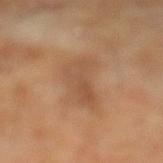<record>
<biopsy_status>not biopsied; imaged during a skin examination</biopsy_status>
<lighting>cross-polarized</lighting>
<site>left lower leg</site>
<lesion_size>
  <long_diameter_mm_approx>5.5</long_diameter_mm_approx>
</lesion_size>
<image>
  <source>total-body photography crop</source>
  <field_of_view_mm>15</field_of_view_mm>
</image>
<automated_metrics>
  <area_mm2_approx>10.0</area_mm2_approx>
  <eccentricity>0.9</eccentricity>
  <shape_asymmetry>0.4</shape_asymmetry>
  <vs_skin_contrast_norm>5.0</vs_skin_contrast_norm>
</automated_metrics>
<patient>
  <sex>male</sex>
  <age_approx>65</age_approx>
</patient>
</record>